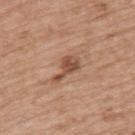Q: Was this lesion biopsied?
A: imaged on a skin check; not biopsied
Q: Who is the patient?
A: male, in their mid-70s
Q: What is the anatomic site?
A: the upper back
Q: What kind of image is this?
A: ~15 mm tile from a whole-body skin photo